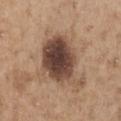patient = male, about 65 years old; imaging modality = ~15 mm crop, total-body skin-cancer survey; location = the chest.